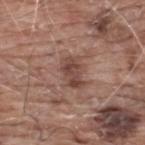Part of a total-body skin-imaging series; this lesion was reviewed on a skin check and was not flagged for biopsy. Automated tile analysis of the lesion measured an area of roughly 6 mm² and a symmetry-axis asymmetry near 0.2. The analysis additionally found a border-irregularity index near 2.5/10, a within-lesion color-variation index near 3.5/10, and a peripheral color-asymmetry measure near 1. Cropped from a whole-body photographic skin survey; the tile spans about 15 mm. A male subject about 60 years old. The lesion's longest dimension is about 3.5 mm. From the upper back. This is a white-light tile.